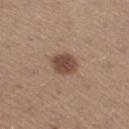Imaged during a routine full-body skin examination; the lesion was not biopsied and no histopathology is available.
Longest diameter approximately 3 mm.
An algorithmic analysis of the crop reported an area of roughly 6.5 mm² and two-axis asymmetry of about 0.15. It also reported a lesion color around L≈46 a*≈19 b*≈25 in CIELAB, a lesion–skin lightness drop of about 13, and a normalized border contrast of about 9.5. The analysis additionally found peripheral color asymmetry of about 1. The software also gave a nevus-likeness score of about 95/100 and a lesion-detection confidence of about 100/100.
A 15 mm close-up tile from a total-body photography series done for melanoma screening.
The lesion is located on the left lower leg.
A female subject, approximately 45 years of age.